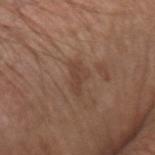biopsy_status: not biopsied; imaged during a skin examination
lighting: white-light
image:
  source: total-body photography crop
  field_of_view_mm: 15
lesion_size:
  long_diameter_mm_approx: 3.5
site: arm
patient:
  sex: male
  age_approx: 70
automated_metrics:
  cielab_L: 42
  cielab_a: 18
  cielab_b: 26
  vs_skin_darker_L: 7.0
  vs_skin_contrast_norm: 5.5
  nevus_likeness_0_100: 0
  lesion_detection_confidence_0_100: 100